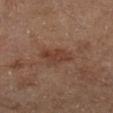Assessment: The lesion was tiled from a total-body skin photograph and was not biopsied. Context: A male patient in their mid-60s. Located on the left lower leg. The tile uses cross-polarized illumination. Cropped from a total-body skin-imaging series; the visible field is about 15 mm. About 3.5 mm across.